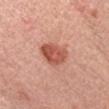Notes:
• workup · catalogued during a skin exam; not biopsied
• imaging modality · 15 mm crop, total-body photography
• lesion diameter · ~4 mm (longest diameter)
• location · the chest
• lighting · white-light
• automated lesion analysis · an area of roughly 9.5 mm²; an average lesion color of about L≈55 a*≈29 b*≈31 (CIELAB), a lesion–skin lightness drop of about 13, and a normalized border contrast of about 8.5
• subject · female, roughly 40 years of age